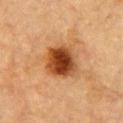A roughly 15 mm field-of-view crop from a total-body skin photograph.
Approximately 4 mm at its widest.
On the chest.
The total-body-photography lesion software estimated a footprint of about 13 mm², an outline eccentricity of about 0.4 (0 = round, 1 = elongated), and two-axis asymmetry of about 0.2. It also reported lesion-presence confidence of about 100/100.
A male subject, in their mid-80s or older.